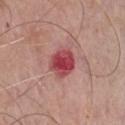{"biopsy_status": "not biopsied; imaged during a skin examination", "image": {"source": "total-body photography crop", "field_of_view_mm": 15}, "site": "chest", "lesion_size": {"long_diameter_mm_approx": 3.5}, "lighting": "white-light", "patient": {"sex": "male", "age_approx": 75}}